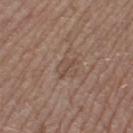location: the left thigh; subject: female, approximately 55 years of age; image source: ~15 mm crop, total-body skin-cancer survey.